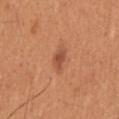Findings:
– workup: no biopsy performed (imaged during a skin exam)